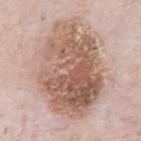Part of a total-body skin-imaging series; this lesion was reviewed on a skin check and was not flagged for biopsy.
This image is a 15 mm lesion crop taken from a total-body photograph.
The subject is a male aged 78 to 82.
The tile uses white-light illumination.
The total-body-photography lesion software estimated a footprint of about 60 mm² and an eccentricity of roughly 0.75. It also reported a lesion color around L≈59 a*≈19 b*≈27 in CIELAB, a lesion–skin lightness drop of about 14, and a normalized border contrast of about 9.
The lesion is on the front of the torso.